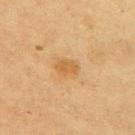| feature | finding |
|---|---|
| workup | total-body-photography surveillance lesion; no biopsy |
| illumination | cross-polarized |
| lesion diameter | ~2.5 mm (longest diameter) |
| image | 15 mm crop, total-body photography |
| body site | the right upper arm |
| subject | female, aged approximately 55 |
| image-analysis metrics | a classifier nevus-likeness of about 30/100 and a lesion-detection confidence of about 100/100 |